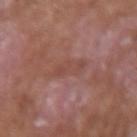Acquisition and patient details: The lesion is located on the right forearm. The subject is a male aged around 65. Cropped from a whole-body photographic skin survey; the tile spans about 15 mm.